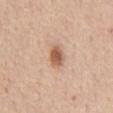biopsy_status: not biopsied; imaged during a skin examination
patient:
  sex: female
  age_approx: 65
image:
  source: total-body photography crop
  field_of_view_mm: 15
site: abdomen